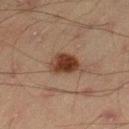follow-up=imaged on a skin check; not biopsied
location=the left thigh
diameter=about 3.5 mm
image source=15 mm crop, total-body photography
illumination=cross-polarized illumination
subject=male, aged 43–47